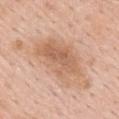Automated tile analysis of the lesion measured a lesion–skin lightness drop of about 9. And it measured a within-lesion color-variation index near 4.5/10 and a peripheral color-asymmetry measure near 1.5. The subject is a male about 55 years old. From the mid back. This image is a 15 mm lesion crop taken from a total-body photograph.